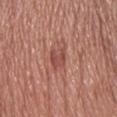The lesion was photographed on a routine skin check and not biopsied; there is no pathology result.
The lesion is on the front of the torso.
The lesion-visualizer software estimated an area of roughly 5 mm², an outline eccentricity of about 0.75 (0 = round, 1 = elongated), and a shape-asymmetry score of about 0.25 (0 = symmetric). The analysis additionally found a border-irregularity rating of about 3/10, internal color variation of about 4 on a 0–10 scale, and radial color variation of about 1.5. The analysis additionally found a nevus-likeness score of about 20/100 and lesion-presence confidence of about 100/100.
A lesion tile, about 15 mm wide, cut from a 3D total-body photograph.
This is a white-light tile.
A male patient, about 75 years old.